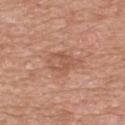site=the mid back | lighting=white-light illumination | patient=male, aged 48–52 | automated metrics=an average lesion color of about L≈55 a*≈22 b*≈31 (CIELAB) and a normalized lesion–skin contrast near 5.5 | imaging modality=~15 mm crop, total-body skin-cancer survey.